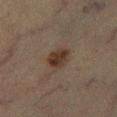Part of a total-body skin-imaging series; this lesion was reviewed on a skin check and was not flagged for biopsy. This is a cross-polarized tile. A region of skin cropped from a whole-body photographic capture, roughly 15 mm wide. The lesion is located on the right lower leg. The subject is a male aged around 65.